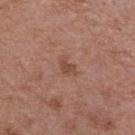Captured during whole-body skin photography for melanoma surveillance; the lesion was not biopsied. A male subject, about 55 years old. On the chest. About 2.5 mm across. Imaged with white-light lighting. The lesion-visualizer software estimated a lesion color around L≈47 a*≈22 b*≈28 in CIELAB, about 8 CIELAB-L* units darker than the surrounding skin, and a normalized border contrast of about 6. A roughly 15 mm field-of-view crop from a total-body skin photograph.